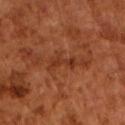  lighting: cross-polarized
  patient:
    sex: male
    age_approx: 65
  image:
    source: total-body photography crop
    field_of_view_mm: 15
  lesion_size:
    long_diameter_mm_approx: 3.5
  automated_metrics:
    area_mm2_approx: 4.5
    shape_asymmetry: 0.4
    border_irregularity_0_10: 5.5
    peripheral_color_asymmetry: 0.0